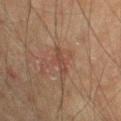Q: What is the imaging modality?
A: ~15 mm tile from a whole-body skin photo
Q: Lesion size?
A: ≈3.5 mm
Q: What are the patient's age and sex?
A: male, approximately 75 years of age
Q: Automated lesion metrics?
A: a shape eccentricity near 0.95; a mean CIELAB color near L≈37 a*≈18 b*≈23 and a normalized border contrast of about 6; a nevus-likeness score of about 0/100 and a detector confidence of about 100 out of 100 that the crop contains a lesion
Q: Where on the body is the lesion?
A: the left upper arm